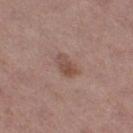Notes:
- biopsy status: total-body-photography surveillance lesion; no biopsy
- lesion size: ~3 mm (longest diameter)
- location: the leg
- patient: female, aged 38–42
- acquisition: total-body-photography crop, ~15 mm field of view